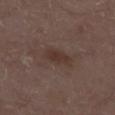No biopsy was performed on this lesion — it was imaged during a full skin examination and was not determined to be concerning. On the left lower leg. A lesion tile, about 15 mm wide, cut from a 3D total-body photograph. A male subject, approximately 70 years of age.